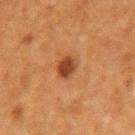Context: Located on the right forearm. Cropped from a total-body skin-imaging series; the visible field is about 15 mm. A female patient, aged 38–42. Automated tile analysis of the lesion measured a footprint of about 5 mm², a shape eccentricity near 0.35, and a symmetry-axis asymmetry near 0.2. The analysis additionally found a mean CIELAB color near L≈37 a*≈24 b*≈33, roughly 11 lightness units darker than nearby skin, and a normalized border contrast of about 9.5. And it measured a classifier nevus-likeness of about 95/100 and a detector confidence of about 100 out of 100 that the crop contains a lesion.